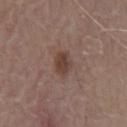Recorded during total-body skin imaging; not selected for excision or biopsy.
A male subject in their 80s.
This is a white-light tile.
The lesion is on the abdomen.
The lesion-visualizer software estimated a footprint of about 6 mm², a shape eccentricity near 0.6, and a symmetry-axis asymmetry near 0.2. And it measured an average lesion color of about L≈41 a*≈17 b*≈23 (CIELAB), a lesion–skin lightness drop of about 9, and a normalized lesion–skin contrast near 7.5. It also reported border irregularity of about 1.5 on a 0–10 scale, internal color variation of about 3 on a 0–10 scale, and peripheral color asymmetry of about 1.
A 15 mm close-up tile from a total-body photography series done for melanoma screening.